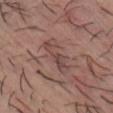Q: Was this lesion biopsied?
A: imaged on a skin check; not biopsied
Q: Lesion location?
A: the chest
Q: What are the patient's age and sex?
A: male, aged 28 to 32
Q: What is the imaging modality?
A: 15 mm crop, total-body photography
Q: Automated lesion metrics?
A: a footprint of about 8 mm², an outline eccentricity of about 0.95 (0 = round, 1 = elongated), and two-axis asymmetry of about 0.3; an automated nevus-likeness rating near 0 out of 100 and a detector confidence of about 60 out of 100 that the crop contains a lesion
Q: What lighting was used for the tile?
A: white-light